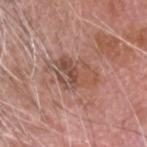{"biopsy_status": "not biopsied; imaged during a skin examination", "site": "head or neck", "lighting": "white-light", "image": {"source": "total-body photography crop", "field_of_view_mm": 15}, "lesion_size": {"long_diameter_mm_approx": 6.0}, "patient": {"sex": "male", "age_approx": 75}}